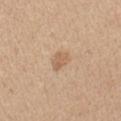Impression: This lesion was catalogued during total-body skin photography and was not selected for biopsy. Acquisition and patient details: A lesion tile, about 15 mm wide, cut from a 3D total-body photograph. A female subject, approximately 45 years of age. The tile uses white-light illumination. The lesion is located on the left upper arm. An algorithmic analysis of the crop reported a footprint of about 4 mm², an eccentricity of roughly 0.75, and two-axis asymmetry of about 0.3. It also reported an average lesion color of about L≈61 a*≈18 b*≈33 (CIELAB), a lesion–skin lightness drop of about 9, and a lesion-to-skin contrast of about 6 (normalized; higher = more distinct). The software also gave a border-irregularity rating of about 3/10. It also reported an automated nevus-likeness rating near 45 out of 100 and lesion-presence confidence of about 100/100.